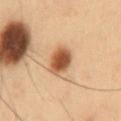Part of a total-body skin-imaging series; this lesion was reviewed on a skin check and was not flagged for biopsy.
The patient is a male aged approximately 55.
An algorithmic analysis of the crop reported an eccentricity of roughly 0.55. It also reported a mean CIELAB color near L≈45 a*≈18 b*≈30, a lesion–skin lightness drop of about 14, and a normalized border contrast of about 10.5. The software also gave a nevus-likeness score of about 100/100 and a detector confidence of about 100 out of 100 that the crop contains a lesion.
A roughly 15 mm field-of-view crop from a total-body skin photograph.
Captured under cross-polarized illumination.
From the abdomen.